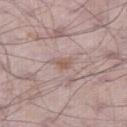Q: Is there a histopathology result?
A: total-body-photography surveillance lesion; no biopsy
Q: How was this image acquired?
A: ~15 mm crop, total-body skin-cancer survey
Q: Lesion location?
A: the right thigh
Q: Who is the patient?
A: male, about 70 years old